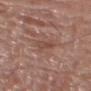No biopsy was performed on this lesion — it was imaged during a full skin examination and was not determined to be concerning.
Imaged with white-light lighting.
Cropped from a whole-body photographic skin survey; the tile spans about 15 mm.
A male subject, roughly 80 years of age.
The lesion is located on the right forearm.